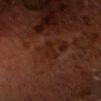Assessment: The lesion was tiled from a total-body skin photograph and was not biopsied. Image and clinical context: A 15 mm crop from a total-body photograph taken for skin-cancer surveillance. The lesion is located on the head or neck. About 3 mm across. Imaged with cross-polarized lighting. The patient is a male in their mid- to late 50s.